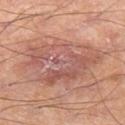Imaged during a routine full-body skin examination; the lesion was not biopsied and no histopathology is available. The patient is a male aged 53 to 57. A lesion tile, about 15 mm wide, cut from a 3D total-body photograph. Captured under cross-polarized illumination. Located on the left thigh.